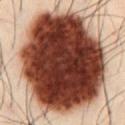Q: Is there a histopathology result?
A: catalogued during a skin exam; not biopsied
Q: What lighting was used for the tile?
A: cross-polarized
Q: What is the lesion's diameter?
A: ≈12.5 mm
Q: What are the patient's age and sex?
A: male, approximately 50 years of age
Q: Automated lesion metrics?
A: an area of roughly 105 mm², an outline eccentricity of about 0.6 (0 = round, 1 = elongated), and a symmetry-axis asymmetry near 0.1; border irregularity of about 1.5 on a 0–10 scale, a color-variation rating of about 9/10, and a peripheral color-asymmetry measure near 3; a nevus-likeness score of about 100/100
Q: How was this image acquired?
A: total-body-photography crop, ~15 mm field of view
Q: Where on the body is the lesion?
A: the front of the torso